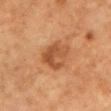The lesion is on the right upper arm. Longest diameter approximately 4 mm. A close-up tile cropped from a whole-body skin photograph, about 15 mm across. The patient is a female approximately 55 years of age. Imaged with cross-polarized lighting.